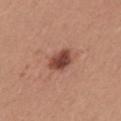Recorded during total-body skin imaging; not selected for excision or biopsy. A region of skin cropped from a whole-body photographic capture, roughly 15 mm wide. On the mid back. The subject is a female aged around 25.